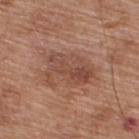No biopsy was performed on this lesion — it was imaged during a full skin examination and was not determined to be concerning.
Located on the upper back.
The total-body-photography lesion software estimated a lesion area of about 15 mm², an outline eccentricity of about 0.75 (0 = round, 1 = elongated), and a symmetry-axis asymmetry near 0.35.
A 15 mm close-up extracted from a 3D total-body photography capture.
A male subject about 65 years old.
Measured at roughly 5.5 mm in maximum diameter.
This is a white-light tile.